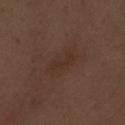{"biopsy_status": "not biopsied; imaged during a skin examination", "site": "right forearm", "lighting": "white-light", "image": {"source": "total-body photography crop", "field_of_view_mm": 15}, "patient": {"sex": "male", "age_approx": 70}}